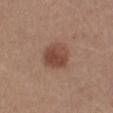This lesion was catalogued during total-body skin photography and was not selected for biopsy. A 15 mm close-up extracted from a 3D total-body photography capture. A female subject roughly 40 years of age. Automated image analysis of the tile measured an eccentricity of roughly 0.35 and a shape-asymmetry score of about 0.15 (0 = symmetric). It also reported a mean CIELAB color near L≈45 a*≈21 b*≈27 and a normalized border contrast of about 8. The analysis additionally found a peripheral color-asymmetry measure near 1.5. Captured under white-light illumination. The lesion is located on the right lower leg.